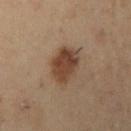Acquisition and patient details: A male subject, aged 53–57. A 15 mm crop from a total-body photograph taken for skin-cancer surveillance. This is a cross-polarized tile. On the right upper arm.